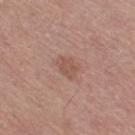{
  "biopsy_status": "not biopsied; imaged during a skin examination",
  "image": {
    "source": "total-body photography crop",
    "field_of_view_mm": 15
  },
  "site": "right thigh",
  "patient": {
    "sex": "male",
    "age_approx": 70
  }
}